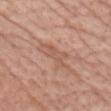Context: A female subject aged around 65. Longest diameter approximately 5 mm. Located on the chest. A lesion tile, about 15 mm wide, cut from a 3D total-body photograph. Captured under white-light illumination.